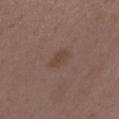Impression:
This lesion was catalogued during total-body skin photography and was not selected for biopsy.
Acquisition and patient details:
The lesion is on the back. The recorded lesion diameter is about 3 mm. This image is a 15 mm lesion crop taken from a total-body photograph. A female patient aged 33 to 37. Imaged with white-light lighting.